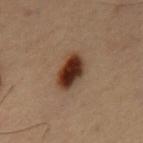| field | value |
|---|---|
| follow-up | total-body-photography surveillance lesion; no biopsy |
| imaging modality | ~15 mm tile from a whole-body skin photo |
| lighting | cross-polarized illumination |
| body site | the mid back |
| automated lesion analysis | an area of roughly 9.5 mm², a shape eccentricity near 0.85, and a shape-asymmetry score of about 0.2 (0 = symmetric); an automated nevus-likeness rating near 100 out of 100 and lesion-presence confidence of about 100/100 |
| patient | male, in their mid-50s |
| size | about 4.5 mm |